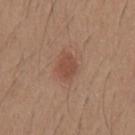The patient is a male about 20 years old. Imaged with white-light lighting. Automated tile analysis of the lesion measured a shape eccentricity near 0.65. It also reported a classifier nevus-likeness of about 95/100. Longest diameter approximately 3 mm. A region of skin cropped from a whole-body photographic capture, roughly 15 mm wide. The lesion is on the mid back.